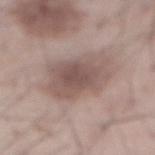This lesion was catalogued during total-body skin photography and was not selected for biopsy. A close-up tile cropped from a whole-body skin photograph, about 15 mm across. The lesion's longest dimension is about 6 mm. The tile uses white-light illumination. From the abdomen. The patient is a male aged 53–57.